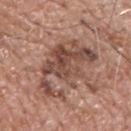<tbp_lesion>
<site>back</site>
<patient>
  <sex>male</sex>
  <age_approx>60</age_approx>
</patient>
<automated_metrics>
  <cielab_L>47</cielab_L>
  <cielab_a>20</cielab_a>
  <cielab_b>26</cielab_b>
  <vs_skin_darker_L>11.0</vs_skin_darker_L>
  <vs_skin_contrast_norm>8.0</vs_skin_contrast_norm>
  <nevus_likeness_0_100>30</nevus_likeness_0_100>
</automated_metrics>
<lesion_size>
  <long_diameter_mm_approx>7.5</long_diameter_mm_approx>
</lesion_size>
<image>
  <source>total-body photography crop</source>
  <field_of_view_mm>15</field_of_view_mm>
</image>
<lighting>white-light</lighting>
</tbp_lesion>